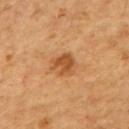Clinical impression:
Captured during whole-body skin photography for melanoma surveillance; the lesion was not biopsied.
Acquisition and patient details:
This image is a 15 mm lesion crop taken from a total-body photograph. A male subject roughly 60 years of age. Captured under cross-polarized illumination. Located on the upper back. Automated image analysis of the tile measured a lesion area of about 5.5 mm², an eccentricity of roughly 0.4, and two-axis asymmetry of about 0.25. It also reported a mean CIELAB color near L≈50 a*≈25 b*≈40 and a normalized lesion–skin contrast near 8. It also reported border irregularity of about 3 on a 0–10 scale and radial color variation of about 0.5. The analysis additionally found a classifier nevus-likeness of about 80/100 and a detector confidence of about 100 out of 100 that the crop contains a lesion.